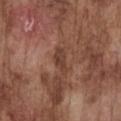biopsy status: no biopsy performed (imaged during a skin exam); image: ~15 mm tile from a whole-body skin photo; body site: the chest; subject: male, about 75 years old.